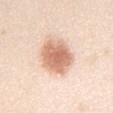Findings:
- biopsy status — imaged on a skin check; not biopsied
- patient — male, aged around 25
- lighting — white-light
- site — the chest
- imaging modality — ~15 mm crop, total-body skin-cancer survey
- diameter — about 5.5 mm